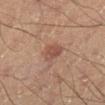Impression: Imaged during a routine full-body skin examination; the lesion was not biopsied and no histopathology is available. Acquisition and patient details: An algorithmic analysis of the crop reported two-axis asymmetry of about 0.3. And it measured a color-variation rating of about 2/10 and radial color variation of about 0.5. Cropped from a total-body skin-imaging series; the visible field is about 15 mm. The lesion is located on the right lower leg. Measured at roughly 2.5 mm in maximum diameter. The subject is a male roughly 40 years of age.